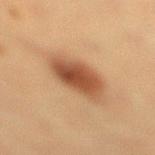Findings:
– biopsy status · no biopsy performed (imaged during a skin exam)
– acquisition · total-body-photography crop, ~15 mm field of view
– subject · female, aged approximately 40
– diameter · about 4.5 mm
– site · the right lower leg
– tile lighting · cross-polarized illumination